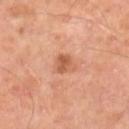notes = no biopsy performed (imaged during a skin exam)
subject = male, aged 48–52
site = the left leg
tile lighting = cross-polarized illumination
image source = ~15 mm tile from a whole-body skin photo
automated metrics = an area of roughly 4.5 mm²; internal color variation of about 2.5 on a 0–10 scale and a peripheral color-asymmetry measure near 1; a nevus-likeness score of about 35/100 and a detector confidence of about 100 out of 100 that the crop contains a lesion
diameter = about 3 mm